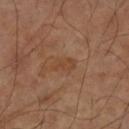<case>
<biopsy_status>not biopsied; imaged during a skin examination</biopsy_status>
<site>right upper arm</site>
<image>
  <source>total-body photography crop</source>
  <field_of_view_mm>15</field_of_view_mm>
</image>
<lesion_size>
  <long_diameter_mm_approx>2.5</long_diameter_mm_approx>
</lesion_size>
<lighting>cross-polarized</lighting>
<patient>
  <sex>male</sex>
  <age_approx>60</age_approx>
</patient>
</case>